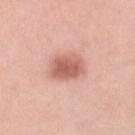| feature | finding |
|---|---|
| follow-up | total-body-photography surveillance lesion; no biopsy |
| TBP lesion metrics | an area of roughly 11 mm², a shape eccentricity near 0.65, and two-axis asymmetry of about 0.2; lesion-presence confidence of about 100/100 |
| body site | the right lower leg |
| patient | female, aged 38–42 |
| illumination | white-light |
| lesion diameter | about 4 mm |
| image | ~15 mm crop, total-body skin-cancer survey |